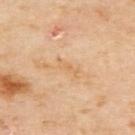Clinical impression: Part of a total-body skin-imaging series; this lesion was reviewed on a skin check and was not flagged for biopsy. Context: A female subject about 60 years old. The lesion is on the upper back. A region of skin cropped from a whole-body photographic capture, roughly 15 mm wide.